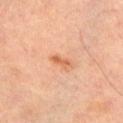Clinical impression:
Captured during whole-body skin photography for melanoma surveillance; the lesion was not biopsied.
Clinical summary:
A lesion tile, about 15 mm wide, cut from a 3D total-body photograph. Measured at roughly 3 mm in maximum diameter. Imaged with cross-polarized lighting. From the front of the torso. A male patient, in their mid-40s.